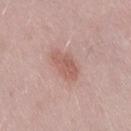follow-up: catalogued during a skin exam; not biopsied | illumination: white-light | patient: male, about 55 years old | anatomic site: the right thigh | size: ~4 mm (longest diameter) | acquisition: ~15 mm crop, total-body skin-cancer survey.